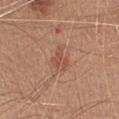biopsy status: no biopsy performed (imaged during a skin exam); size: ≈3 mm; acquisition: 15 mm crop, total-body photography; subject: female, in their mid- to late 30s; lighting: white-light; site: the upper back.